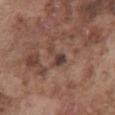workup: total-body-photography surveillance lesion; no biopsy | image: ~15 mm tile from a whole-body skin photo | body site: the abdomen | subject: male, aged around 75 | illumination: white-light | size: about 3 mm.